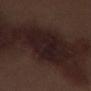Imaged during a routine full-body skin examination; the lesion was not biopsied and no histopathology is available. The lesion is located on the left thigh. Automated image analysis of the tile measured an outline eccentricity of about 0.75 (0 = round, 1 = elongated) and two-axis asymmetry of about 0.2. It also reported a nevus-likeness score of about 0/100 and a detector confidence of about 50 out of 100 that the crop contains a lesion. A male subject, aged 68 to 72. Longest diameter approximately 6.5 mm. Imaged with white-light lighting. A lesion tile, about 15 mm wide, cut from a 3D total-body photograph.